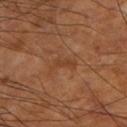workup: no biopsy performed (imaged during a skin exam)
lighting: cross-polarized illumination
subject: male, aged 58–62
lesion size: about 3 mm
anatomic site: the left lower leg
image source: ~15 mm tile from a whole-body skin photo